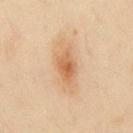The lesion was tiled from a total-body skin photograph and was not biopsied. This is a cross-polarized tile. A male patient in their 50s. Cropped from a total-body skin-imaging series; the visible field is about 15 mm. The lesion is located on the front of the torso.